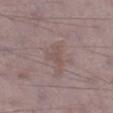Captured during whole-body skin photography for melanoma surveillance; the lesion was not biopsied.
Automated tile analysis of the lesion measured a shape eccentricity near 0.75 and a symmetry-axis asymmetry near 0.45. And it measured a lesion color around L≈50 a*≈16 b*≈20 in CIELAB, roughly 6 lightness units darker than nearby skin, and a lesion-to-skin contrast of about 4.5 (normalized; higher = more distinct). The software also gave a border-irregularity index near 4.5/10, internal color variation of about 1 on a 0–10 scale, and peripheral color asymmetry of about 0.
Located on the right lower leg.
This image is a 15 mm lesion crop taken from a total-body photograph.
A male patient, aged 68–72.
The lesion's longest dimension is about 3 mm.
The tile uses white-light illumination.